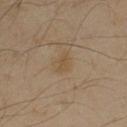Clinical impression: Recorded during total-body skin imaging; not selected for excision or biopsy. Acquisition and patient details: The tile uses cross-polarized illumination. Automated tile analysis of the lesion measured an area of roughly 2.5 mm², an eccentricity of roughly 0.85, and a symmetry-axis asymmetry near 0.35. The software also gave about 6 CIELAB-L* units darker than the surrounding skin and a lesion-to-skin contrast of about 5.5 (normalized; higher = more distinct). The analysis additionally found a color-variation rating of about 0/10 and a peripheral color-asymmetry measure near 0. The software also gave a classifier nevus-likeness of about 0/100. A male patient in their 50s. A roughly 15 mm field-of-view crop from a total-body skin photograph. Approximately 2.5 mm at its widest. The lesion is located on the right upper arm.